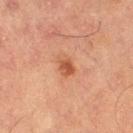Imaged during a routine full-body skin examination; the lesion was not biopsied and no histopathology is available.
A 15 mm close-up tile from a total-body photography series done for melanoma screening.
Approximately 3 mm at its widest.
The lesion is located on the left thigh.
A male patient aged around 65.